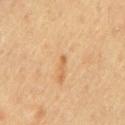{"biopsy_status": "not biopsied; imaged during a skin examination", "image": {"source": "total-body photography crop", "field_of_view_mm": 15}, "patient": {"sex": "male", "age_approx": 60}, "lighting": "cross-polarized", "automated_metrics": {"cielab_L": 57, "cielab_a": 20, "cielab_b": 38, "vs_skin_darker_L": 8.0, "vs_skin_contrast_norm": 6.0}, "site": "chest", "lesion_size": {"long_diameter_mm_approx": 2.0}}